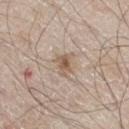This lesion was catalogued during total-body skin photography and was not selected for biopsy.
Cropped from a total-body skin-imaging series; the visible field is about 15 mm.
About 3 mm across.
The tile uses white-light illumination.
A male patient, aged 58 to 62.
On the right thigh.